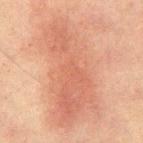• workup — total-body-photography surveillance lesion; no biopsy
• lighting — cross-polarized
• lesion diameter — ~12.5 mm (longest diameter)
• patient — male, aged around 65
• anatomic site — the back
• image source — ~15 mm tile from a whole-body skin photo